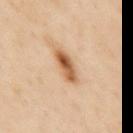Q: What lighting was used for the tile?
A: cross-polarized
Q: Patient demographics?
A: male, aged 53–57
Q: Lesion size?
A: about 4 mm
Q: What is the anatomic site?
A: the upper back
Q: What is the imaging modality?
A: ~15 mm tile from a whole-body skin photo
Q: What did automated image analysis measure?
A: a lesion color around L≈59 a*≈21 b*≈38 in CIELAB, roughly 15 lightness units darker than nearby skin, and a normalized lesion–skin contrast near 10; border irregularity of about 2.5 on a 0–10 scale, internal color variation of about 7.5 on a 0–10 scale, and a peripheral color-asymmetry measure near 3